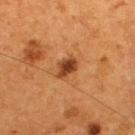Assessment:
Imaged during a routine full-body skin examination; the lesion was not biopsied and no histopathology is available.
Clinical summary:
Cropped from a total-body skin-imaging series; the visible field is about 15 mm. Located on the upper back. A male subject aged around 55. This is a cross-polarized tile.